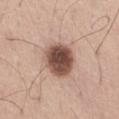{"biopsy_status": "not biopsied; imaged during a skin examination", "site": "right thigh", "lesion_size": {"long_diameter_mm_approx": 4.0}, "automated_metrics": {"cielab_L": 50, "cielab_a": 19, "cielab_b": 25, "vs_skin_darker_L": 19.0, "vs_skin_contrast_norm": 12.5, "border_irregularity_0_10": 1.0, "color_variation_0_10": 6.0, "peripheral_color_asymmetry": 1.5}, "patient": {"sex": "male", "age_approx": 55}, "image": {"source": "total-body photography crop", "field_of_view_mm": 15}}